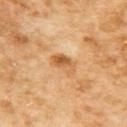Q: How was the tile lit?
A: cross-polarized
Q: Who is the patient?
A: female, aged 58–62
Q: What is the lesion's diameter?
A: about 3.5 mm
Q: Automated lesion metrics?
A: border irregularity of about 3 on a 0–10 scale, a within-lesion color-variation index near 8/10, and a peripheral color-asymmetry measure near 3
Q: What is the imaging modality?
A: ~15 mm crop, total-body skin-cancer survey
Q: Lesion location?
A: the upper back